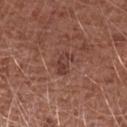Part of a total-body skin-imaging series; this lesion was reviewed on a skin check and was not flagged for biopsy. A male patient, in their mid-40s. A region of skin cropped from a whole-body photographic capture, roughly 15 mm wide. On the arm.